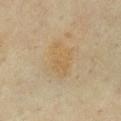Assessment:
The lesion was tiled from a total-body skin photograph and was not biopsied.
Background:
From the chest. The subject is a female aged around 60. About 5 mm across. The total-body-photography lesion software estimated a footprint of about 12 mm², a shape eccentricity near 0.75, and a shape-asymmetry score of about 0.3 (0 = symmetric). The software also gave an average lesion color of about L≈55 a*≈11 b*≈34 (CIELAB), a lesion–skin lightness drop of about 5, and a normalized lesion–skin contrast near 5. The analysis additionally found internal color variation of about 2.5 on a 0–10 scale. The analysis additionally found an automated nevus-likeness rating near 0 out of 100 and a detector confidence of about 100 out of 100 that the crop contains a lesion. This image is a 15 mm lesion crop taken from a total-body photograph. Captured under cross-polarized illumination.